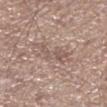Recorded during total-body skin imaging; not selected for excision or biopsy. The tile uses white-light illumination. Cropped from a total-body skin-imaging series; the visible field is about 15 mm. The subject is a male roughly 75 years of age. The lesion is on the left lower leg. About 4.5 mm across. Automated image analysis of the tile measured roughly 7 lightness units darker than nearby skin. The software also gave border irregularity of about 7 on a 0–10 scale, a within-lesion color-variation index near 2/10, and radial color variation of about 0.5.